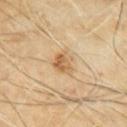{
  "biopsy_status": "not biopsied; imaged during a skin examination",
  "patient": {
    "sex": "male",
    "age_approx": 60
  },
  "site": "mid back",
  "image": {
    "source": "total-body photography crop",
    "field_of_view_mm": 15
  },
  "lighting": "cross-polarized",
  "automated_metrics": {
    "cielab_L": 59,
    "cielab_a": 17,
    "cielab_b": 37,
    "vs_skin_darker_L": 10.0,
    "nevus_likeness_0_100": 55
  },
  "lesion_size": {
    "long_diameter_mm_approx": 3.0
  }
}